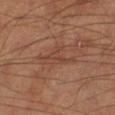Case summary:
• notes · imaged on a skin check; not biopsied
• lesion size · ~4 mm (longest diameter)
• subject · male, aged 63–67
• anatomic site · the left lower leg
• acquisition · 15 mm crop, total-body photography